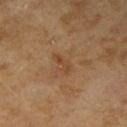Assessment: The lesion was photographed on a routine skin check and not biopsied; there is no pathology result.